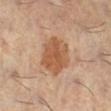Q: Is there a histopathology result?
A: imaged on a skin check; not biopsied
Q: How large is the lesion?
A: ≈4.5 mm
Q: What are the patient's age and sex?
A: female, aged approximately 60
Q: Automated lesion metrics?
A: radial color variation of about 1; a classifier nevus-likeness of about 40/100 and a lesion-detection confidence of about 100/100
Q: Lesion location?
A: the leg
Q: How was this image acquired?
A: ~15 mm tile from a whole-body skin photo